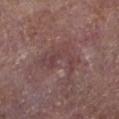– subject · male, roughly 80 years of age
– image-analysis metrics · a footprint of about 9 mm² and a symmetry-axis asymmetry near 0.65; a mean CIELAB color near L≈41 a*≈20 b*≈17, roughly 6 lightness units darker than nearby skin, and a lesion-to-skin contrast of about 5 (normalized; higher = more distinct); a border-irregularity index near 10/10, a color-variation rating of about 1.5/10, and a peripheral color-asymmetry measure near 0.5
– image source · ~15 mm crop, total-body skin-cancer survey
– body site · the left lower leg